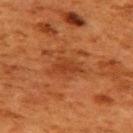subject: female, in their 50s | lighting: cross-polarized illumination | diameter: ≈3.5 mm | body site: the upper back | acquisition: 15 mm crop, total-body photography.